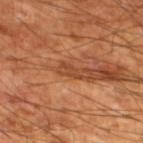Captured during whole-body skin photography for melanoma surveillance; the lesion was not biopsied. Imaged with cross-polarized lighting. A 15 mm crop from a total-body photograph taken for skin-cancer surveillance. The subject is a male aged approximately 60. The lesion is on the left lower leg. The recorded lesion diameter is about 2.5 mm.